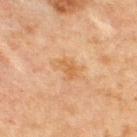Image and clinical context:
Cropped from a whole-body photographic skin survey; the tile spans about 15 mm. The lesion is on the chest. Captured under cross-polarized illumination. Longest diameter approximately 2.5 mm. The lesion-visualizer software estimated border irregularity of about 3.5 on a 0–10 scale, internal color variation of about 1 on a 0–10 scale, and peripheral color asymmetry of about 0.5. The analysis additionally found a nevus-likeness score of about 0/100 and a lesion-detection confidence of about 100/100. A male patient approximately 65 years of age.